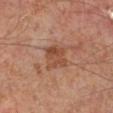* workup — no biopsy performed (imaged during a skin exam)
* location — the left leg
* size — ≈3 mm
* automated lesion analysis — a footprint of about 7.5 mm², an outline eccentricity of about 0.45 (0 = round, 1 = elongated), and two-axis asymmetry of about 0.15; an average lesion color of about L≈47 a*≈22 b*≈32 (CIELAB), roughly 9 lightness units darker than nearby skin, and a lesion-to-skin contrast of about 7 (normalized; higher = more distinct); a classifier nevus-likeness of about 0/100 and a lesion-detection confidence of about 100/100
* image — 15 mm crop, total-body photography
* illumination — cross-polarized
* subject — male, in their 50s